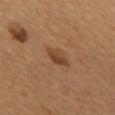notes: total-body-photography surveillance lesion; no biopsy | acquisition: ~15 mm crop, total-body skin-cancer survey | lesion size: about 3 mm | site: the back | illumination: cross-polarized | subject: male, aged approximately 55.